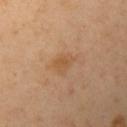A female patient about 40 years old. A roughly 15 mm field-of-view crop from a total-body skin photograph. The lesion is located on the arm. Imaged with cross-polarized lighting.